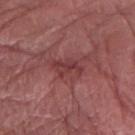This lesion was catalogued during total-body skin photography and was not selected for biopsy. Approximately 3.5 mm at its widest. A male patient, about 75 years old. A region of skin cropped from a whole-body photographic capture, roughly 15 mm wide. Imaged with white-light lighting. From the right forearm.